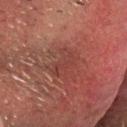{
  "biopsy_status": "not biopsied; imaged during a skin examination",
  "image": {
    "source": "total-body photography crop",
    "field_of_view_mm": 15
  },
  "site": "head or neck",
  "lesion_size": {
    "long_diameter_mm_approx": 3.5
  },
  "patient": {
    "sex": "male",
    "age_approx": 60
  },
  "automated_metrics": {
    "eccentricity": 0.8,
    "shape_asymmetry": 0.45,
    "vs_skin_darker_L": 4.0,
    "color_variation_0_10": 2.0,
    "peripheral_color_asymmetry": 0.5
  },
  "lighting": "cross-polarized"
}